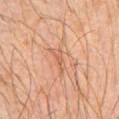Context: From the chest. Cropped from a whole-body photographic skin survey; the tile spans about 15 mm. A male patient, approximately 30 years of age.